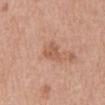Q: Illumination type?
A: white-light
Q: How large is the lesion?
A: about 2.5 mm
Q: What are the patient's age and sex?
A: male, aged 68 to 72
Q: Where on the body is the lesion?
A: the abdomen
Q: How was this image acquired?
A: ~15 mm crop, total-body skin-cancer survey